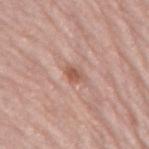<tbp_lesion>
  <patient>
    <sex>female</sex>
    <age_approx>65</age_approx>
  </patient>
  <site>mid back</site>
  <image>
    <source>total-body photography crop</source>
    <field_of_view_mm>15</field_of_view_mm>
  </image>
</tbp_lesion>